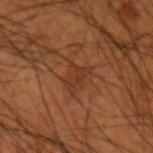Assessment: Recorded during total-body skin imaging; not selected for excision or biopsy. Image and clinical context: A close-up tile cropped from a whole-body skin photograph, about 15 mm across. On the left upper arm. The tile uses cross-polarized illumination. About 2.5 mm across. A male patient aged 48–52.